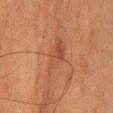This lesion was catalogued during total-body skin photography and was not selected for biopsy.
The tile uses cross-polarized illumination.
The subject is a female in their 60s.
Longest diameter approximately 6 mm.
A roughly 15 mm field-of-view crop from a total-body skin photograph.
The lesion is located on the head or neck.